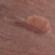This lesion was catalogued during total-body skin photography and was not selected for biopsy. A female patient, aged 33 to 37. Cropped from a whole-body photographic skin survey; the tile spans about 15 mm. The lesion is on the left thigh.